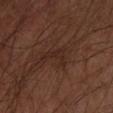patient: male, aged around 60
illumination: cross-polarized illumination
size: about 3.5 mm
acquisition: 15 mm crop, total-body photography
location: the left forearm
automated metrics: border irregularity of about 8 on a 0–10 scale, internal color variation of about 0 on a 0–10 scale, and a peripheral color-asymmetry measure near 0